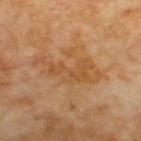Notes:
* biopsy status: catalogued during a skin exam; not biopsied
* anatomic site: the upper back
* patient: male, approximately 70 years of age
* image source: ~15 mm crop, total-body skin-cancer survey